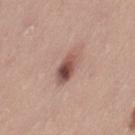Q: Was a biopsy performed?
A: no biopsy performed (imaged during a skin exam)
Q: How large is the lesion?
A: about 4 mm
Q: What lighting was used for the tile?
A: white-light
Q: What is the imaging modality?
A: 15 mm crop, total-body photography
Q: Lesion location?
A: the left thigh
Q: Who is the patient?
A: female, about 35 years old